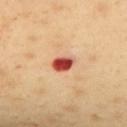  biopsy_status: not biopsied; imaged during a skin examination
  lighting: cross-polarized
  patient:
    sex: male
    age_approx: 45
  automated_metrics:
    vs_skin_darker_L: 21.0
    vs_skin_contrast_norm: 13.5
    color_variation_0_10: 4.5
    peripheral_color_asymmetry: 1.5
    nevus_likeness_0_100: 0
    lesion_detection_confidence_0_100: 100
  site: mid back
  image:
    source: total-body photography crop
    field_of_view_mm: 15
  lesion_size:
    long_diameter_mm_approx: 3.0